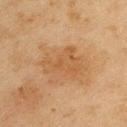Captured during whole-body skin photography for melanoma surveillance; the lesion was not biopsied. The total-body-photography lesion software estimated two-axis asymmetry of about 0.55. The analysis additionally found a border-irregularity index near 6.5/10 and a within-lesion color-variation index near 2/10. A male patient, roughly 45 years of age. On the left upper arm. A close-up tile cropped from a whole-body skin photograph, about 15 mm across. Imaged with cross-polarized lighting. The lesion's longest dimension is about 4.5 mm.